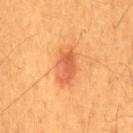anatomic site — the chest
patient — male, aged around 60
imaging modality — ~15 mm crop, total-body skin-cancer survey